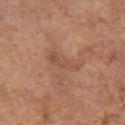Impression:
This lesion was catalogued during total-body skin photography and was not selected for biopsy.
Background:
A female subject aged 63 to 67. A close-up tile cropped from a whole-body skin photograph, about 15 mm across. Located on the left upper arm.